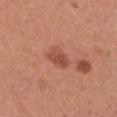{
  "lesion_size": {
    "long_diameter_mm_approx": 3.0
  },
  "image": {
    "source": "total-body photography crop",
    "field_of_view_mm": 15
  },
  "patient": {
    "sex": "female",
    "age_approx": 25
  },
  "lighting": "white-light",
  "site": "left upper arm",
  "automated_metrics": {
    "area_mm2_approx": 4.5,
    "eccentricity": 0.8,
    "shape_asymmetry": 0.25,
    "lesion_detection_confidence_0_100": 100
  }
}